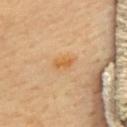This lesion was catalogued during total-body skin photography and was not selected for biopsy. On the upper back. A 15 mm close-up tile from a total-body photography series done for melanoma screening. This is a cross-polarized tile. About 3 mm across. A female patient aged 68–72. The lesion-visualizer software estimated an outline eccentricity of about 0.75 (0 = round, 1 = elongated). The analysis additionally found lesion-presence confidence of about 100/100.